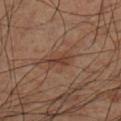Captured during whole-body skin photography for melanoma surveillance; the lesion was not biopsied. The lesion-visualizer software estimated a shape eccentricity near 0.85 and a symmetry-axis asymmetry near 0.4. The analysis additionally found a lesion color around L≈37 a*≈19 b*≈26 in CIELAB, about 8 CIELAB-L* units darker than the surrounding skin, and a normalized border contrast of about 7. The software also gave a border-irregularity index near 4/10 and a within-lesion color-variation index near 2.5/10. It also reported a classifier nevus-likeness of about 5/100. A close-up tile cropped from a whole-body skin photograph, about 15 mm across. This is a cross-polarized tile. The lesion is located on the left lower leg. The subject is a male aged around 60. About 3 mm across.